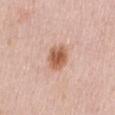Findings:
- follow-up · catalogued during a skin exam; not biopsied
- lighting · white-light illumination
- image source · ~15 mm tile from a whole-body skin photo
- patient · female, approximately 50 years of age
- image-analysis metrics · a lesion color around L≈59 a*≈23 b*≈32 in CIELAB and a normalized lesion–skin contrast near 9.5; an automated nevus-likeness rating near 95 out of 100
- body site · the mid back